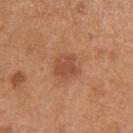| feature | finding |
|---|---|
| workup | imaged on a skin check; not biopsied |